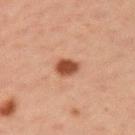Q: Patient demographics?
A: female, approximately 40 years of age
Q: Lesion size?
A: about 2.5 mm
Q: How was this image acquired?
A: total-body-photography crop, ~15 mm field of view
Q: Lesion location?
A: the left upper arm